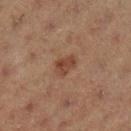biopsy status: imaged on a skin check; not biopsied | patient: female, about 40 years old | diameter: ~3 mm (longest diameter) | site: the leg | tile lighting: cross-polarized illumination | image source: ~15 mm crop, total-body skin-cancer survey.